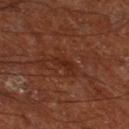| key | value |
|---|---|
| biopsy status | catalogued during a skin exam; not biopsied |
| image-analysis metrics | a lesion color around L≈25 a*≈22 b*≈27 in CIELAB and a normalized border contrast of about 6; a border-irregularity rating of about 3.5/10, internal color variation of about 0.5 on a 0–10 scale, and peripheral color asymmetry of about 0; a classifier nevus-likeness of about 0/100 and lesion-presence confidence of about 95/100 |
| acquisition | total-body-photography crop, ~15 mm field of view |
| patient | male, about 65 years old |
| site | the right thigh |